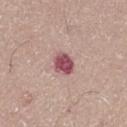biopsy_status: not biopsied; imaged during a skin examination
lesion_size:
  long_diameter_mm_approx: 3.0
patient:
  sex: male
  age_approx: 65
automated_metrics:
  lesion_detection_confidence_0_100: 100
site: left thigh
image:
  source: total-body photography crop
  field_of_view_mm: 15
lighting: white-light